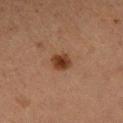This lesion was catalogued during total-body skin photography and was not selected for biopsy.
A lesion tile, about 15 mm wide, cut from a 3D total-body photograph.
A female patient in their 40s.
The lesion's longest dimension is about 2.5 mm.
From the left forearm.